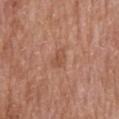Impression:
Imaged during a routine full-body skin examination; the lesion was not biopsied and no histopathology is available.
Context:
A 15 mm crop from a total-body photograph taken for skin-cancer surveillance. Located on the chest. Imaged with white-light lighting. The lesion's longest dimension is about 2.5 mm. A male patient, aged around 65.